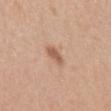Impression: Recorded during total-body skin imaging; not selected for excision or biopsy. Image and clinical context: The lesion is on the left upper arm. The recorded lesion diameter is about 2.5 mm. A female subject, in their mid-20s. Cropped from a total-body skin-imaging series; the visible field is about 15 mm.